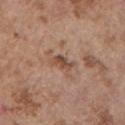Clinical impression:
Part of a total-body skin-imaging series; this lesion was reviewed on a skin check and was not flagged for biopsy.